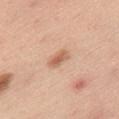workup — total-body-photography surveillance lesion; no biopsy | size — about 2.5 mm | site — the lower back | illumination — white-light illumination | imaging modality — 15 mm crop, total-body photography | subject — male, aged approximately 50.